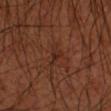The lesion was tiled from a total-body skin photograph and was not biopsied. From the left forearm. A male patient aged approximately 60. About 3 mm across. A 15 mm close-up extracted from a 3D total-body photography capture.